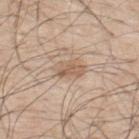No biopsy was performed on this lesion — it was imaged during a full skin examination and was not determined to be concerning.
Located on the upper back.
Approximately 3.5 mm at its widest.
Imaged with white-light lighting.
A male subject about 70 years old.
An algorithmic analysis of the crop reported an area of roughly 6 mm² and a shape-asymmetry score of about 0.2 (0 = symmetric). And it measured a color-variation rating of about 3/10 and peripheral color asymmetry of about 1.
A roughly 15 mm field-of-view crop from a total-body skin photograph.